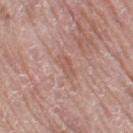Q: Automated lesion metrics?
A: roughly 6 lightness units darker than nearby skin; a border-irregularity index near 2.5/10 and a within-lesion color-variation index near 2.5/10
Q: Where on the body is the lesion?
A: the left thigh
Q: What kind of image is this?
A: 15 mm crop, total-body photography
Q: How was the tile lit?
A: white-light
Q: Patient demographics?
A: female, aged approximately 60